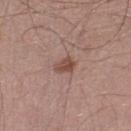Notes:
* biopsy status — imaged on a skin check; not biopsied
* automated metrics — an average lesion color of about L≈48 a*≈20 b*≈25 (CIELAB), about 10 CIELAB-L* units darker than the surrounding skin, and a normalized border contrast of about 7.5; a peripheral color-asymmetry measure near 1
* acquisition — ~15 mm tile from a whole-body skin photo
* patient — male, roughly 60 years of age
* location — the left lower leg
* illumination — white-light
* diameter — ≈2.5 mm